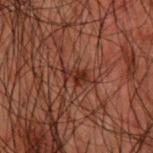Located on the upper back. A male subject, approximately 50 years of age. Cropped from a whole-body photographic skin survey; the tile spans about 15 mm. Imaged with cross-polarized lighting. Measured at roughly 3 mm in maximum diameter.